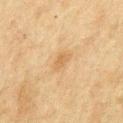| feature | finding |
|---|---|
| lesion diameter | ≈2.5 mm |
| acquisition | ~15 mm crop, total-body skin-cancer survey |
| patient | male, in their mid- to late 70s |
| anatomic site | the chest |
| automated lesion analysis | a shape eccentricity near 0.8 and a shape-asymmetry score of about 0.35 (0 = symmetric); border irregularity of about 3.5 on a 0–10 scale, internal color variation of about 1.5 on a 0–10 scale, and peripheral color asymmetry of about 0.5; an automated nevus-likeness rating near 10 out of 100 |